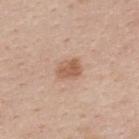Recorded during total-body skin imaging; not selected for excision or biopsy. An algorithmic analysis of the crop reported a footprint of about 5.5 mm², a shape eccentricity near 0.65, and a symmetry-axis asymmetry near 0.25. Located on the upper back. A 15 mm close-up tile from a total-body photography series done for melanoma screening. This is a white-light tile. The subject is a female in their mid-50s.